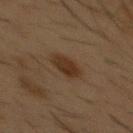{"biopsy_status": "not biopsied; imaged during a skin examination", "patient": {"sex": "male", "age_approx": 55}, "lighting": "cross-polarized", "image": {"source": "total-body photography crop", "field_of_view_mm": 15}, "site": "chest", "automated_metrics": {"area_mm2_approx": 7.0, "eccentricity": 0.65, "shape_asymmetry": 0.15, "cielab_L": 27, "cielab_a": 14, "cielab_b": 25, "vs_skin_contrast_norm": 8.5}}